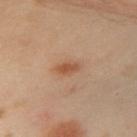Notes:
• biopsy status: total-body-photography surveillance lesion; no biopsy
• image source: total-body-photography crop, ~15 mm field of view
• patient: female, approximately 40 years of age
• lesion size: about 3 mm
• body site: the left upper arm
• illumination: cross-polarized illumination